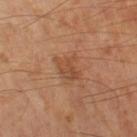Captured during whole-body skin photography for melanoma surveillance; the lesion was not biopsied. A lesion tile, about 15 mm wide, cut from a 3D total-body photograph. A male patient aged approximately 65. This is a cross-polarized tile. The lesion is located on the left thigh.